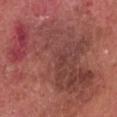Cropped from a total-body skin-imaging series; the visible field is about 15 mm. A male patient approximately 80 years of age. The tile uses white-light illumination. Measured at roughly 16 mm in maximum diameter. Automated image analysis of the tile measured a mean CIELAB color near L≈44 a*≈27 b*≈24. The software also gave a nevus-likeness score of about 0/100 and lesion-presence confidence of about 100/100. The lesion is located on the head or neck. Biopsy histopathology demonstrated a squamous cell carcinoma in situ (malignant).